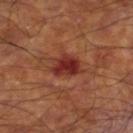Assessment: Imaged during a routine full-body skin examination; the lesion was not biopsied and no histopathology is available. Clinical summary: A male subject, about 60 years old. The recorded lesion diameter is about 3.5 mm. A lesion tile, about 15 mm wide, cut from a 3D total-body photograph. On the right lower leg.